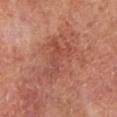Impression: Part of a total-body skin-imaging series; this lesion was reviewed on a skin check and was not flagged for biopsy. Image and clinical context: A male subject, approximately 70 years of age. A close-up tile cropped from a whole-body skin photograph, about 15 mm across. The lesion is located on the left lower leg.